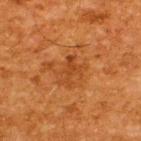biopsy status=no biopsy performed (imaged during a skin exam)
subject=male, approximately 65 years of age
illumination=cross-polarized
TBP lesion metrics=an average lesion color of about L≈37 a*≈25 b*≈37 (CIELAB), a lesion–skin lightness drop of about 7, and a normalized border contrast of about 6; a border-irregularity index near 7/10 and radial color variation of about 0
image=~15 mm tile from a whole-body skin photo
location=the upper back
lesion size=≈3.5 mm